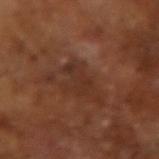No biopsy was performed on this lesion — it was imaged during a full skin examination and was not determined to be concerning.
The lesion-visualizer software estimated a lesion color around L≈30 a*≈20 b*≈26 in CIELAB and a lesion-to-skin contrast of about 6 (normalized; higher = more distinct). It also reported a border-irregularity rating of about 5.5/10. It also reported lesion-presence confidence of about 85/100.
Captured under cross-polarized illumination.
A 15 mm close-up tile from a total-body photography series done for melanoma screening.
The subject is a male in their mid-60s.
The recorded lesion diameter is about 3.5 mm.